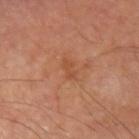<case>
<site>left upper arm</site>
<lighting>cross-polarized</lighting>
<patient>
  <sex>male</sex>
  <age_approx>70</age_approx>
</patient>
<lesion_size>
  <long_diameter_mm_approx>3.0</long_diameter_mm_approx>
</lesion_size>
<image>
  <source>total-body photography crop</source>
  <field_of_view_mm>15</field_of_view_mm>
</image>
</case>